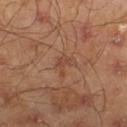<record>
<biopsy_status>not biopsied; imaged during a skin examination</biopsy_status>
<image>
  <source>total-body photography crop</source>
  <field_of_view_mm>15</field_of_view_mm>
</image>
<patient>
  <sex>male</sex>
  <age_approx>45</age_approx>
</patient>
<site>leg</site>
<lesion_size>
  <long_diameter_mm_approx>2.5</long_diameter_mm_approx>
</lesion_size>
</record>